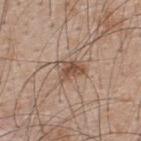workup — catalogued during a skin exam; not biopsied | diameter — about 3.5 mm | location — the upper back | lighting — white-light illumination | acquisition — ~15 mm crop, total-body skin-cancer survey | patient — male, aged 48 to 52.